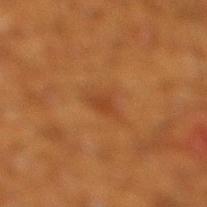biopsy status: total-body-photography surveillance lesion; no biopsy.